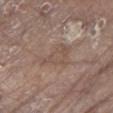No biopsy was performed on this lesion — it was imaged during a full skin examination and was not determined to be concerning. The patient is a male aged 78 to 82. A close-up tile cropped from a whole-body skin photograph, about 15 mm across. Located on the left lower leg. The lesion-visualizer software estimated a within-lesion color-variation index near 3/10. Measured at roughly 5 mm in maximum diameter.